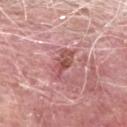No biopsy was performed on this lesion — it was imaged during a full skin examination and was not determined to be concerning.
About 4.5 mm across.
A close-up tile cropped from a whole-body skin photograph, about 15 mm across.
The subject is a male in their 60s.
On the head or neck.
Captured under white-light illumination.